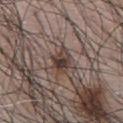This lesion was catalogued during total-body skin photography and was not selected for biopsy. A 15 mm close-up tile from a total-body photography series done for melanoma screening. The subject is a male aged 68–72. Located on the chest.